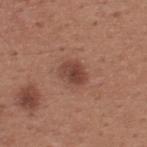<record>
<biopsy_status>not biopsied; imaged during a skin examination</biopsy_status>
<patient>
  <sex>male</sex>
  <age_approx>65</age_approx>
</patient>
<lesion_size>
  <long_diameter_mm_approx>3.0</long_diameter_mm_approx>
</lesion_size>
<lighting>white-light</lighting>
<image>
  <source>total-body photography crop</source>
  <field_of_view_mm>15</field_of_view_mm>
</image>
<site>upper back</site>
</record>